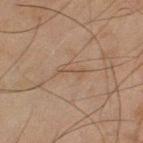| key | value |
|---|---|
| workup | imaged on a skin check; not biopsied |
| body site | the left thigh |
| subject | male, in their mid- to late 40s |
| imaging modality | ~15 mm crop, total-body skin-cancer survey |